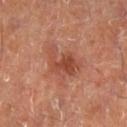Recorded during total-body skin imaging; not selected for excision or biopsy.
From the right lower leg.
Captured under cross-polarized illumination.
This image is a 15 mm lesion crop taken from a total-body photograph.
A male patient, about 50 years old.
Measured at roughly 4.5 mm in maximum diameter.